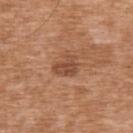{"biopsy_status": "not biopsied; imaged during a skin examination", "patient": {"sex": "male", "age_approx": 75}, "site": "upper back", "image": {"source": "total-body photography crop", "field_of_view_mm": 15}}